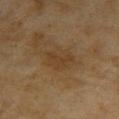Part of a total-body skin-imaging series; this lesion was reviewed on a skin check and was not flagged for biopsy.
A region of skin cropped from a whole-body photographic capture, roughly 15 mm wide.
On the right upper arm.
A female patient aged approximately 60.
Automated tile analysis of the lesion measured a shape eccentricity near 0.7. The analysis additionally found a border-irregularity index near 2.5/10.
The lesion's longest dimension is about 4 mm.